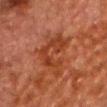No biopsy was performed on this lesion — it was imaged during a full skin examination and was not determined to be concerning.
A male patient about 80 years old.
A 15 mm close-up extracted from a 3D total-body photography capture.
The tile uses cross-polarized illumination.
The lesion is located on the head or neck.
The recorded lesion diameter is about 6.5 mm.